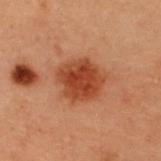A female patient in their 40s.
Automated tile analysis of the lesion measured an average lesion color of about L≈36 a*≈25 b*≈31 (CIELAB). And it measured border irregularity of about 2 on a 0–10 scale and internal color variation of about 3.5 on a 0–10 scale.
A roughly 15 mm field-of-view crop from a total-body skin photograph.
The lesion is on the upper back.